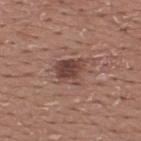Assessment: Recorded during total-body skin imaging; not selected for excision or biopsy. Background: The subject is a male aged 18–22. A lesion tile, about 15 mm wide, cut from a 3D total-body photograph. The recorded lesion diameter is about 4 mm. This is a white-light tile. The lesion-visualizer software estimated internal color variation of about 4 on a 0–10 scale and peripheral color asymmetry of about 1.5. Located on the upper back.